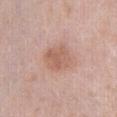biopsy_status: not biopsied; imaged during a skin examination
site: right thigh
lighting: white-light
patient:
  sex: female
  age_approx: 60
image:
  source: total-body photography crop
  field_of_view_mm: 15
automated_metrics:
  border_irregularity_0_10: 1.5
  color_variation_0_10: 3.0
  peripheral_color_asymmetry: 1.0
  lesion_detection_confidence_0_100: 100
lesion_size:
  long_diameter_mm_approx: 3.5